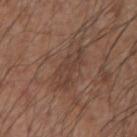Clinical impression: The lesion was photographed on a routine skin check and not biopsied; there is no pathology result. Background: A 15 mm close-up extracted from a 3D total-body photography capture. A male subject about 60 years old. The lesion is located on the left upper arm. The tile uses white-light illumination.